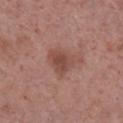Assessment:
The lesion was tiled from a total-body skin photograph and was not biopsied.